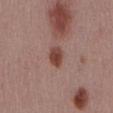No biopsy was performed on this lesion — it was imaged during a full skin examination and was not determined to be concerning. This image is a 15 mm lesion crop taken from a total-body photograph. Automated image analysis of the tile measured an area of roughly 4.5 mm² and a shape eccentricity near 0.7. It also reported a border-irregularity index near 2/10, internal color variation of about 3.5 on a 0–10 scale, and peripheral color asymmetry of about 1. And it measured an automated nevus-likeness rating near 95 out of 100 and a detector confidence of about 100 out of 100 that the crop contains a lesion. Longest diameter approximately 2.5 mm. The subject is a male in their mid-50s. On the mid back.